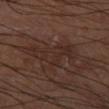| feature | finding |
|---|---|
| notes | imaged on a skin check; not biopsied |
| illumination | cross-polarized |
| image source | ~15 mm crop, total-body skin-cancer survey |
| patient | male, roughly 60 years of age |
| location | the leg |